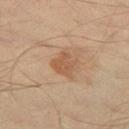Clinical impression: Part of a total-body skin-imaging series; this lesion was reviewed on a skin check and was not flagged for biopsy. Acquisition and patient details: A close-up tile cropped from a whole-body skin photograph, about 15 mm across. The tile uses cross-polarized illumination. Longest diameter approximately 3 mm. The patient is a male roughly 40 years of age. On the left thigh.